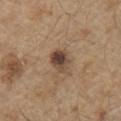The subject is a male approximately 70 years of age.
A 15 mm close-up tile from a total-body photography series done for melanoma screening.
Located on the chest.
The tile uses white-light illumination.